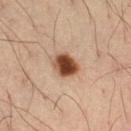The lesion was photographed on a routine skin check and not biopsied; there is no pathology result.
This image is a 15 mm lesion crop taken from a total-body photograph.
This is a cross-polarized tile.
The total-body-photography lesion software estimated a lesion area of about 7 mm², an eccentricity of roughly 0.6, and a shape-asymmetry score of about 0.15 (0 = symmetric). The analysis additionally found a border-irregularity index near 1.5/10 and radial color variation of about 2.
A male subject, about 35 years old.
From the right thigh.
The lesion's longest dimension is about 3 mm.